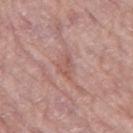Recorded during total-body skin imaging; not selected for excision or biopsy. This is a white-light tile. The subject is a female aged approximately 75. Automated image analysis of the tile measured two-axis asymmetry of about 0.35. The software also gave an average lesion color of about L≈55 a*≈23 b*≈24 (CIELAB), a lesion–skin lightness drop of about 7, and a normalized lesion–skin contrast near 5. The software also gave a color-variation rating of about 2.5/10. It also reported an automated nevus-likeness rating near 0 out of 100. On the left thigh. Measured at roughly 3 mm in maximum diameter. A roughly 15 mm field-of-view crop from a total-body skin photograph.